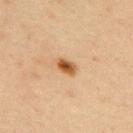Notes:
* notes: catalogued during a skin exam; not biopsied
* location: the upper back
* subject: female, aged approximately 45
* acquisition: total-body-photography crop, ~15 mm field of view